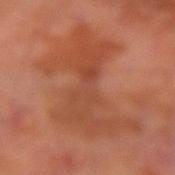Captured during whole-body skin photography for melanoma surveillance; the lesion was not biopsied.
Captured under cross-polarized illumination.
The lesion is on the right forearm.
Measured at roughly 11.5 mm in maximum diameter.
Automated tile analysis of the lesion measured border irregularity of about 8 on a 0–10 scale and radial color variation of about 2. The software also gave an automated nevus-likeness rating near 0 out of 100 and lesion-presence confidence of about 100/100.
A 15 mm close-up tile from a total-body photography series done for melanoma screening.
A male subject, roughly 70 years of age.